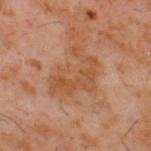Q: Is there a histopathology result?
A: no biopsy performed (imaged during a skin exam)
Q: What did automated image analysis measure?
A: an area of roughly 15 mm² and a symmetry-axis asymmetry near 0.5; border irregularity of about 8.5 on a 0–10 scale, internal color variation of about 3 on a 0–10 scale, and a peripheral color-asymmetry measure near 1; an automated nevus-likeness rating near 0 out of 100
Q: Who is the patient?
A: male, aged 58–62
Q: What is the imaging modality?
A: total-body-photography crop, ~15 mm field of view
Q: Illumination type?
A: cross-polarized
Q: What is the lesion's diameter?
A: ≈5.5 mm
Q: Where on the body is the lesion?
A: the upper back